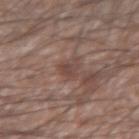patient = male, in their mid- to late 60s; image = ~15 mm crop, total-body skin-cancer survey; location = the left forearm.